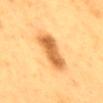{
  "biopsy_status": "not biopsied; imaged during a skin examination",
  "patient": {
    "sex": "male",
    "age_approx": 60
  },
  "lighting": "cross-polarized",
  "image": {
    "source": "total-body photography crop",
    "field_of_view_mm": 15
  },
  "site": "mid back"
}